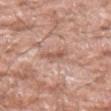* workup: total-body-photography surveillance lesion; no biopsy
* location: the left forearm
* imaging modality: total-body-photography crop, ~15 mm field of view
* patient: male, aged approximately 60